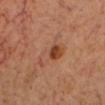Findings:
- follow-up — total-body-photography surveillance lesion; no biopsy
- tile lighting — cross-polarized
- TBP lesion metrics — a border-irregularity rating of about 4.5/10, internal color variation of about 9 on a 0–10 scale, and a peripheral color-asymmetry measure near 2.5; a classifier nevus-likeness of about 80/100 and a detector confidence of about 100 out of 100 that the crop contains a lesion
- patient — male, aged approximately 70
- site — the head or neck
- image — ~15 mm tile from a whole-body skin photo
- lesion size — ~4.5 mm (longest diameter)